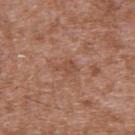| feature | finding |
|---|---|
| notes | total-body-photography surveillance lesion; no biopsy |
| lesion diameter | ~2.5 mm (longest diameter) |
| subject | male, about 45 years old |
| acquisition | 15 mm crop, total-body photography |
| anatomic site | the upper back |
| tile lighting | white-light |